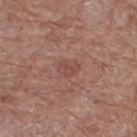• workup — no biopsy performed (imaged during a skin exam)
• size — ~3 mm (longest diameter)
• tile lighting — white-light
• acquisition — ~15 mm crop, total-body skin-cancer survey
• site — the leg
• subject — female, in their 80s
• TBP lesion metrics — an area of roughly 4 mm² and an outline eccentricity of about 0.8 (0 = round, 1 = elongated); a border-irregularity rating of about 2.5/10 and a peripheral color-asymmetry measure near 1; an automated nevus-likeness rating near 0 out of 100 and a detector confidence of about 100 out of 100 that the crop contains a lesion